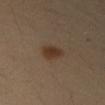<record>
<biopsy_status>not biopsied; imaged during a skin examination</biopsy_status>
</record>